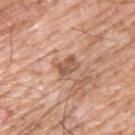workup: total-body-photography surveillance lesion; no biopsy | site: the upper back | subject: male, roughly 60 years of age | lighting: white-light | lesion size: ~3 mm (longest diameter) | imaging modality: total-body-photography crop, ~15 mm field of view.